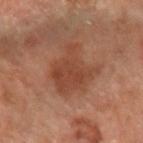workup: imaged on a skin check; not biopsied | site: the left forearm | image-analysis metrics: a lesion area of about 18 mm² and a shape-asymmetry score of about 0.35 (0 = symmetric); roughly 7 lightness units darker than nearby skin and a normalized lesion–skin contrast near 6.5; border irregularity of about 4.5 on a 0–10 scale | subject: roughly 70 years of age | imaging modality: ~15 mm tile from a whole-body skin photo.